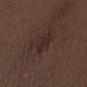Imaged during a routine full-body skin examination; the lesion was not biopsied and no histopathology is available.